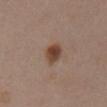This lesion was catalogued during total-body skin photography and was not selected for biopsy. A female subject in their mid-50s. This is a white-light tile. On the chest. A 15 mm close-up extracted from a 3D total-body photography capture. The recorded lesion diameter is about 3 mm.